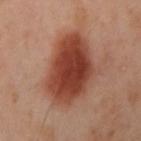<record>
<biopsy_status>not biopsied; imaged during a skin examination</biopsy_status>
<image>
  <source>total-body photography crop</source>
  <field_of_view_mm>15</field_of_view_mm>
</image>
<site>left arm</site>
<patient>
  <sex>female</sex>
  <age_approx>40</age_approx>
</patient>
<automated_metrics>
  <area_mm2_approx>32.0</area_mm2_approx>
  <eccentricity>0.75</eccentricity>
  <border_irregularity_0_10>2.5</border_irregularity_0_10>
  <color_variation_0_10>5.5</color_variation_0_10>
  <peripheral_color_asymmetry>1.5</peripheral_color_asymmetry>
  <nevus_likeness_0_100>100</nevus_likeness_0_100>
  <lesion_detection_confidence_0_100>100</lesion_detection_confidence_0_100>
</automated_metrics>
</record>